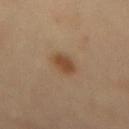Captured during whole-body skin photography for melanoma surveillance; the lesion was not biopsied. The lesion is on the lower back. The tile uses cross-polarized illumination. A female subject approximately 60 years of age. A roughly 15 mm field-of-view crop from a total-body skin photograph. The lesion-visualizer software estimated a mean CIELAB color near L≈47 a*≈18 b*≈33, roughly 10 lightness units darker than nearby skin, and a normalized lesion–skin contrast near 8.